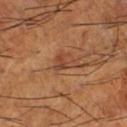Notes:
- notes · total-body-photography surveillance lesion; no biopsy
- imaging modality · ~15 mm crop, total-body skin-cancer survey
- patient · male, aged 63–67
- diameter · about 3 mm
- lighting · cross-polarized illumination
- site · the left lower leg